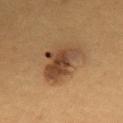patient:
  sex: female
  age_approx: 30
lighting: cross-polarized
image:
  source: total-body photography crop
  field_of_view_mm: 15
automated_metrics:
  cielab_L: 41
  cielab_a: 17
  cielab_b: 30
  vs_skin_darker_L: 11.0
  vs_skin_contrast_norm: 9.5
  border_irregularity_0_10: 4.0
  color_variation_0_10: 6.0
  peripheral_color_asymmetry: 2.0
  lesion_detection_confidence_0_100: 100
site: upper back
lesion_size:
  long_diameter_mm_approx: 6.0